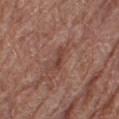{"site": "leg", "lighting": "white-light", "automated_metrics": {"area_mm2_approx": 3.0, "eccentricity": 0.9, "shape_asymmetry": 0.35, "border_irregularity_0_10": 4.0, "peripheral_color_asymmetry": 0.0}, "image": {"source": "total-body photography crop", "field_of_view_mm": 15}, "patient": {"sex": "female", "age_approx": 80}}